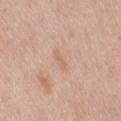<case>
  <biopsy_status>not biopsied; imaged during a skin examination</biopsy_status>
  <patient>
    <sex>male</sex>
    <age_approx>75</age_approx>
  </patient>
  <lighting>white-light</lighting>
  <image>
    <source>total-body photography crop</source>
    <field_of_view_mm>15</field_of_view_mm>
  </image>
  <automated_metrics>
    <vs_skin_darker_L>6.0</vs_skin_darker_L>
    <vs_skin_contrast_norm>4.5</vs_skin_contrast_norm>
    <nevus_likeness_0_100>0</nevus_likeness_0_100>
    <lesion_detection_confidence_0_100>100</lesion_detection_confidence_0_100>
  </automated_metrics>
  <lesion_size>
    <long_diameter_mm_approx>2.5</long_diameter_mm_approx>
  </lesion_size>
  <site>back</site>
</case>